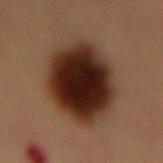Captured during whole-body skin photography for melanoma surveillance; the lesion was not biopsied. This is a cross-polarized tile. Approximately 8 mm at its widest. On the front of the torso. A male patient approximately 55 years of age. A roughly 15 mm field-of-view crop from a total-body skin photograph.